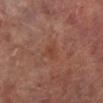Imaged during a routine full-body skin examination; the lesion was not biopsied and no histopathology is available.
The lesion-visualizer software estimated an area of roughly 4.5 mm², an outline eccentricity of about 0.55 (0 = round, 1 = elongated), and a shape-asymmetry score of about 0.5 (0 = symmetric). The software also gave an automated nevus-likeness rating near 0 out of 100 and a lesion-detection confidence of about 100/100.
The patient is a male aged 63 to 67.
A 15 mm close-up extracted from a 3D total-body photography capture.
Longest diameter approximately 3 mm.
The lesion is located on the left lower leg.